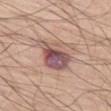workup: catalogued during a skin exam; not biopsied | acquisition: 15 mm crop, total-body photography | patient: male, approximately 70 years of age | site: the left thigh | size: about 5 mm | lighting: white-light.